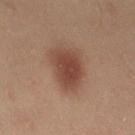No biopsy was performed on this lesion — it was imaged during a full skin examination and was not determined to be concerning. The patient is a female aged 38 to 42. The lesion-visualizer software estimated a footprint of about 14 mm², an outline eccentricity of about 0.7 (0 = round, 1 = elongated), and a symmetry-axis asymmetry near 0.2. The software also gave a mean CIELAB color near L≈35 a*≈17 b*≈22, about 9 CIELAB-L* units darker than the surrounding skin, and a normalized lesion–skin contrast near 8.5. The software also gave an automated nevus-likeness rating near 100 out of 100 and lesion-presence confidence of about 100/100. This image is a 15 mm lesion crop taken from a total-body photograph. The lesion is located on the right thigh.